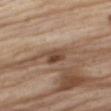{"biopsy_status": "not biopsied; imaged during a skin examination", "site": "right thigh", "lighting": "white-light", "automated_metrics": {"cielab_L": 46, "cielab_a": 18, "cielab_b": 28, "vs_skin_darker_L": 13.0, "nevus_likeness_0_100": 20}, "image": {"source": "total-body photography crop", "field_of_view_mm": 15}, "patient": {"sex": "male", "age_approx": 70}}